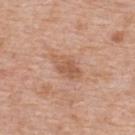Recorded during total-body skin imaging; not selected for excision or biopsy.